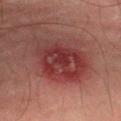The lesion was photographed on a routine skin check and not biopsied; there is no pathology result. The recorded lesion diameter is about 6.5 mm. This image is a 15 mm lesion crop taken from a total-body photograph. The lesion is on the leg. A male subject approximately 75 years of age. An algorithmic analysis of the crop reported a lesion area of about 27 mm², a shape eccentricity near 0.65, and a symmetry-axis asymmetry near 0.2. It also reported a classifier nevus-likeness of about 0/100. Imaged with cross-polarized lighting.